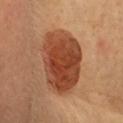Notes:
- workup: imaged on a skin check; not biopsied
- location: the head or neck
- imaging modality: 15 mm crop, total-body photography
- patient: female, roughly 60 years of age
- size: ~8 mm (longest diameter)
- tile lighting: cross-polarized